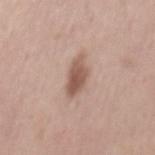This lesion was catalogued during total-body skin photography and was not selected for biopsy.
The lesion-visualizer software estimated a border-irregularity rating of about 3/10, internal color variation of about 3 on a 0–10 scale, and radial color variation of about 1.
A male patient, in their mid-40s.
This is a white-light tile.
The lesion is on the mid back.
Measured at roughly 4.5 mm in maximum diameter.
A 15 mm close-up tile from a total-body photography series done for melanoma screening.